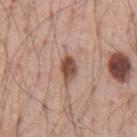biopsy_status: not biopsied; imaged during a skin examination
lighting: white-light
patient:
  sex: male
  age_approx: 55
lesion_size:
  long_diameter_mm_approx: 3.0
site: chest
image:
  source: total-body photography crop
  field_of_view_mm: 15